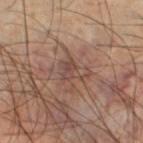Notes:
– patient · male, in their 50s
– site · the left lower leg
– tile lighting · cross-polarized
– imaging modality · ~15 mm tile from a whole-body skin photo